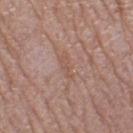Findings:
– notes: total-body-photography surveillance lesion; no biopsy
– anatomic site: the right thigh
– lesion diameter: ~3 mm (longest diameter)
– acquisition: total-body-photography crop, ~15 mm field of view
– patient: female, aged approximately 55